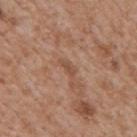site — the mid back; patient — male, in their mid- to late 60s; imaging modality — ~15 mm crop, total-body skin-cancer survey.